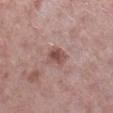This lesion was catalogued during total-body skin photography and was not selected for biopsy. Longest diameter approximately 2.5 mm. On the right lower leg. A female subject, in their 50s. This image is a 15 mm lesion crop taken from a total-body photograph. An algorithmic analysis of the crop reported an area of roughly 4.5 mm². The analysis additionally found a nevus-likeness score of about 35/100 and a lesion-detection confidence of about 100/100. Captured under white-light illumination.